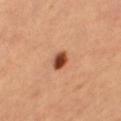Imaged with cross-polarized lighting. The subject is a female approximately 70 years of age. Longest diameter approximately 2.5 mm. The lesion is located on the right thigh. The lesion-visualizer software estimated a lesion area of about 4 mm², a shape eccentricity near 0.7, and two-axis asymmetry of about 0.15. The software also gave a border-irregularity rating of about 1.5/10, a color-variation rating of about 4/10, and a peripheral color-asymmetry measure near 1. The software also gave a classifier nevus-likeness of about 100/100 and a detector confidence of about 100 out of 100 that the crop contains a lesion. A lesion tile, about 15 mm wide, cut from a 3D total-body photograph.